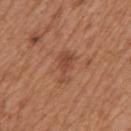Part of a total-body skin-imaging series; this lesion was reviewed on a skin check and was not flagged for biopsy.
Longest diameter approximately 4 mm.
Cropped from a total-body skin-imaging series; the visible field is about 15 mm.
On the mid back.
Imaged with white-light lighting.
A male subject, aged approximately 65.